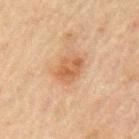This image is a 15 mm lesion crop taken from a total-body photograph.
The patient is a male in their mid- to late 40s.
From the left upper arm.